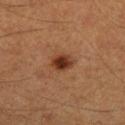Case summary:
* workup: catalogued during a skin exam; not biopsied
* anatomic site: the leg
* lesion size: about 2.5 mm
* illumination: cross-polarized illumination
* imaging modality: 15 mm crop, total-body photography
* patient: male, about 60 years old
* automated lesion analysis: a color-variation rating of about 4/10 and radial color variation of about 1